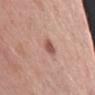The lesion was photographed on a routine skin check and not biopsied; there is no pathology result.
The lesion-visualizer software estimated about 7 CIELAB-L* units darker than the surrounding skin and a lesion-to-skin contrast of about 5 (normalized; higher = more distinct). And it measured a classifier nevus-likeness of about 60/100 and lesion-presence confidence of about 100/100.
The subject is a male roughly 55 years of age.
From the right upper arm.
A 15 mm close-up tile from a total-body photography series done for melanoma screening.
The recorded lesion diameter is about 4 mm.
This is a white-light tile.